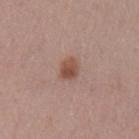Captured during whole-body skin photography for melanoma surveillance; the lesion was not biopsied. A lesion tile, about 15 mm wide, cut from a 3D total-body photograph. Located on the left upper arm. This is a white-light tile. A female subject about 45 years old. The lesion's longest dimension is about 2.5 mm.